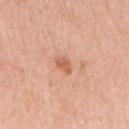Findings:
– follow-up: no biopsy performed (imaged during a skin exam)
– site: the right upper arm
– image source: total-body-photography crop, ~15 mm field of view
– size: ≈2.5 mm
– lighting: white-light
– patient: female, in their mid-60s